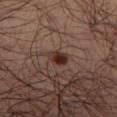Assessment:
The lesion was photographed on a routine skin check and not biopsied; there is no pathology result.
Image and clinical context:
A male subject, aged approximately 35. A 15 mm close-up extracted from a 3D total-body photography capture. The lesion is located on the left lower leg. The recorded lesion diameter is about 2.5 mm.